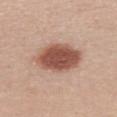Q: Is there a histopathology result?
A: catalogued during a skin exam; not biopsied
Q: Who is the patient?
A: female, about 20 years old
Q: What is the lesion's diameter?
A: about 6.5 mm
Q: What is the anatomic site?
A: the back
Q: What did automated image analysis measure?
A: about 16 CIELAB-L* units darker than the surrounding skin and a lesion-to-skin contrast of about 10.5 (normalized; higher = more distinct); a border-irregularity rating of about 1.5/10, a within-lesion color-variation index near 4/10, and radial color variation of about 1; a classifier nevus-likeness of about 100/100 and a detector confidence of about 100 out of 100 that the crop contains a lesion
Q: How was this image acquired?
A: total-body-photography crop, ~15 mm field of view
Q: What lighting was used for the tile?
A: white-light illumination